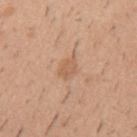Image and clinical context: A male subject, aged 38–42. About 2.5 mm across. This is a white-light tile. A 15 mm close-up tile from a total-body photography series done for melanoma screening. Located on the mid back.